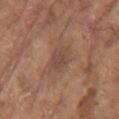biopsy_status: not biopsied; imaged during a skin examination
lighting: white-light
site: left upper arm
lesion_size:
  long_diameter_mm_approx: 3.5
patient:
  sex: male
  age_approx: 80
image:
  source: total-body photography crop
  field_of_view_mm: 15